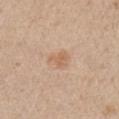<case>
  <biopsy_status>not biopsied; imaged during a skin examination</biopsy_status>
  <image>
    <source>total-body photography crop</source>
    <field_of_view_mm>15</field_of_view_mm>
  </image>
  <patient>
    <sex>male</sex>
    <age_approx>75</age_approx>
  </patient>
  <lighting>white-light</lighting>
  <lesion_size>
    <long_diameter_mm_approx>2.5</long_diameter_mm_approx>
  </lesion_size>
  <site>right upper arm</site>
</case>